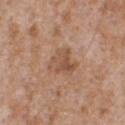Assessment: Imaged during a routine full-body skin examination; the lesion was not biopsied and no histopathology is available. Image and clinical context: The subject is a male aged approximately 65. A lesion tile, about 15 mm wide, cut from a 3D total-body photograph. On the front of the torso.